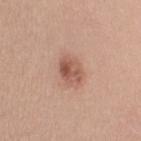Captured during whole-body skin photography for melanoma surveillance; the lesion was not biopsied. The recorded lesion diameter is about 3.5 mm. A female subject, aged 28 to 32. An algorithmic analysis of the crop reported a footprint of about 6.5 mm² and two-axis asymmetry of about 0.2. The analysis additionally found a border-irregularity index near 2/10 and a within-lesion color-variation index near 6.5/10. And it measured lesion-presence confidence of about 100/100. A region of skin cropped from a whole-body photographic capture, roughly 15 mm wide. The tile uses white-light illumination. On the left upper arm.